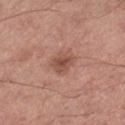body site = the left thigh | patient = male, about 65 years old | image source = ~15 mm tile from a whole-body skin photo.